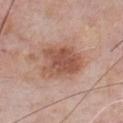Clinical impression:
The lesion was tiled from a total-body skin photograph and was not biopsied.
Image and clinical context:
From the chest. A male patient, about 60 years old. A close-up tile cropped from a whole-body skin photograph, about 15 mm across. The lesion-visualizer software estimated a lesion area of about 17 mm², an outline eccentricity of about 0.65 (0 = round, 1 = elongated), and a symmetry-axis asymmetry near 0.25. And it measured an average lesion color of about L≈53 a*≈22 b*≈29 (CIELAB), a lesion–skin lightness drop of about 12, and a normalized lesion–skin contrast near 8. It also reported a border-irregularity rating of about 3/10, a within-lesion color-variation index near 4/10, and radial color variation of about 1.5. The software also gave a classifier nevus-likeness of about 40/100 and a detector confidence of about 100 out of 100 that the crop contains a lesion. Imaged with white-light lighting.